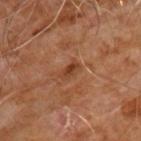From the chest.
Captured under cross-polarized illumination.
The recorded lesion diameter is about 2.5 mm.
The subject is a male roughly 60 years of age.
Cropped from a total-body skin-imaging series; the visible field is about 15 mm.